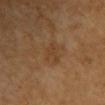subject: female, in their mid-50s; acquisition: 15 mm crop, total-body photography; location: the chest.